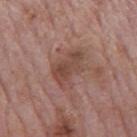Imaged during a routine full-body skin examination; the lesion was not biopsied and no histopathology is available.
A male patient, aged around 75.
On the mid back.
Measured at roughly 5 mm in maximum diameter.
A lesion tile, about 15 mm wide, cut from a 3D total-body photograph.